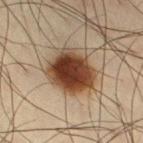Imaged during a routine full-body skin examination; the lesion was not biopsied and no histopathology is available. Automated tile analysis of the lesion measured a lesion-to-skin contrast of about 14.5 (normalized; higher = more distinct). The software also gave a classifier nevus-likeness of about 100/100 and a lesion-detection confidence of about 100/100. The patient is a male aged 33–37. The lesion is on the leg. A 15 mm close-up extracted from a 3D total-body photography capture.